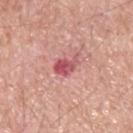Case summary:
* subject — male, aged around 70
* location — the upper back
* image source — total-body-photography crop, ~15 mm field of view
* tile lighting — white-light illumination
* diameter — about 3.5 mm
* TBP lesion metrics — a lesion area of about 5 mm² and a shape-asymmetry score of about 0.35 (0 = symmetric); a color-variation rating of about 4.5/10 and a peripheral color-asymmetry measure near 1.5